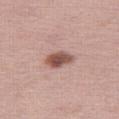Acquisition and patient details: A roughly 15 mm field-of-view crop from a total-body skin photograph. Automated image analysis of the tile measured a nevus-likeness score of about 85/100 and a lesion-detection confidence of about 100/100. Imaged with white-light lighting. A female patient aged 38–42. Located on the leg.